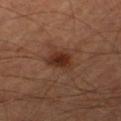Q: Was this lesion biopsied?
A: total-body-photography surveillance lesion; no biopsy
Q: Lesion location?
A: the leg
Q: How was the tile lit?
A: cross-polarized
Q: Who is the patient?
A: male, aged around 65
Q: How large is the lesion?
A: about 3 mm
Q: How was this image acquired?
A: total-body-photography crop, ~15 mm field of view